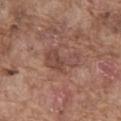This lesion was catalogued during total-body skin photography and was not selected for biopsy. The patient is a male roughly 75 years of age. Located on the abdomen. This image is a 15 mm lesion crop taken from a total-body photograph. The tile uses white-light illumination.